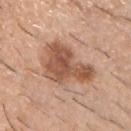Image and clinical context:
Automated image analysis of the tile measured roughly 13 lightness units darker than nearby skin and a normalized lesion–skin contrast near 9. The software also gave radial color variation of about 1.5. The tile uses white-light illumination. Cropped from a whole-body photographic skin survey; the tile spans about 15 mm. Located on the front of the torso. Longest diameter approximately 7 mm. A male patient about 60 years old.